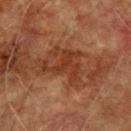Captured under cross-polarized illumination.
The total-body-photography lesion software estimated an area of roughly 9 mm², a shape eccentricity near 0.65, and two-axis asymmetry of about 0.7. The software also gave a lesion color around L≈30 a*≈22 b*≈28 in CIELAB, roughly 7 lightness units darker than nearby skin, and a normalized lesion–skin contrast near 7. And it measured a border-irregularity rating of about 10/10. It also reported a nevus-likeness score of about 0/100 and a lesion-detection confidence of about 100/100.
A roughly 15 mm field-of-view crop from a total-body skin photograph.
About 4.5 mm across.
On the left upper arm.
A male subject, roughly 75 years of age.